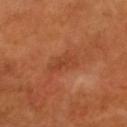<record>
<biopsy_status>not biopsied; imaged during a skin examination</biopsy_status>
<lesion_size>
  <long_diameter_mm_approx>3.5</long_diameter_mm_approx>
</lesion_size>
<image>
  <source>total-body photography crop</source>
  <field_of_view_mm>15</field_of_view_mm>
</image>
<patient>
  <sex>male</sex>
  <age_approx>60</age_approx>
</patient>
<site>head or neck</site>
</record>